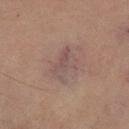Assessment: This lesion was catalogued during total-body skin photography and was not selected for biopsy. Background: A roughly 15 mm field-of-view crop from a total-body skin photograph. The recorded lesion diameter is about 4 mm. Automated image analysis of the tile measured an area of roughly 8.5 mm², an eccentricity of roughly 0.7, and two-axis asymmetry of about 0.4. The software also gave a lesion color around L≈43 a*≈15 b*≈18 in CIELAB, a lesion–skin lightness drop of about 5, and a normalized lesion–skin contrast near 5.5. It also reported a border-irregularity rating of about 5/10, internal color variation of about 4 on a 0–10 scale, and a peripheral color-asymmetry measure near 1.5. The software also gave a classifier nevus-likeness of about 0/100 and a detector confidence of about 95 out of 100 that the crop contains a lesion. A female subject approximately 70 years of age. Located on the right thigh.